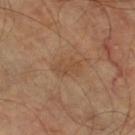Clinical impression:
No biopsy was performed on this lesion — it was imaged during a full skin examination and was not determined to be concerning.
Clinical summary:
A close-up tile cropped from a whole-body skin photograph, about 15 mm across. Imaged with cross-polarized lighting. The subject is in their mid-60s. From the left thigh. About 3.5 mm across. The lesion-visualizer software estimated a lesion–skin lightness drop of about 6. It also reported an automated nevus-likeness rating near 0 out of 100 and a lesion-detection confidence of about 100/100.